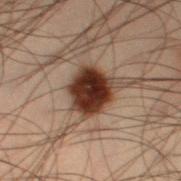follow-up — imaged on a skin check; not biopsied
body site — the leg
acquisition — total-body-photography crop, ~15 mm field of view
lighting — cross-polarized
size — ≈4 mm
patient — male, aged around 55